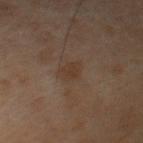<record>
<biopsy_status>not biopsied; imaged during a skin examination</biopsy_status>
<lighting>cross-polarized</lighting>
<image>
  <source>total-body photography crop</source>
  <field_of_view_mm>15</field_of_view_mm>
</image>
<automated_metrics>
  <area_mm2_approx>3.5</area_mm2_approx>
  <eccentricity>0.8</eccentricity>
  <shape_asymmetry>0.2</shape_asymmetry>
  <lesion_detection_confidence_0_100>100</lesion_detection_confidence_0_100>
</automated_metrics>
<lesion_size>
  <long_diameter_mm_approx>3.0</long_diameter_mm_approx>
</lesion_size>
<patient>
  <sex>male</sex>
  <age_approx>70</age_approx>
</patient>
<site>chest</site>
</record>